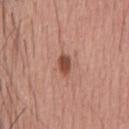The lesion was tiled from a total-body skin photograph and was not biopsied.
Located on the upper back.
Longest diameter approximately 2.5 mm.
This image is a 15 mm lesion crop taken from a total-body photograph.
Captured under white-light illumination.
A male patient, approximately 45 years of age.
Automated tile analysis of the lesion measured an average lesion color of about L≈48 a*≈25 b*≈29 (CIELAB) and a normalized lesion–skin contrast near 10. It also reported border irregularity of about 2.5 on a 0–10 scale, a within-lesion color-variation index near 3.5/10, and radial color variation of about 1.5.